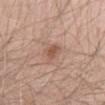The lesion was tiled from a total-body skin photograph and was not biopsied.
Measured at roughly 2.5 mm in maximum diameter.
From the abdomen.
This is a white-light tile.
A male subject aged approximately 50.
A 15 mm close-up extracted from a 3D total-body photography capture.